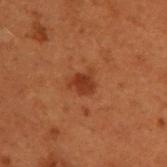* biopsy status: catalogued during a skin exam; not biopsied
* site: the back
* patient: male, aged around 50
* lesion diameter: ~3 mm (longest diameter)
* automated lesion analysis: a footprint of about 5.5 mm² and a shape-asymmetry score of about 0.3 (0 = symmetric); a mean CIELAB color near L≈30 a*≈23 b*≈29 and about 7 CIELAB-L* units darker than the surrounding skin; a border-irregularity rating of about 3/10, a within-lesion color-variation index near 2/10, and a peripheral color-asymmetry measure near 0.5; a nevus-likeness score of about 80/100
* lighting: cross-polarized illumination
* image source: ~15 mm tile from a whole-body skin photo